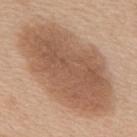| key | value |
|---|---|
| follow-up | no biopsy performed (imaged during a skin exam) |
| location | the mid back |
| tile lighting | white-light |
| diameter | about 13.5 mm |
| acquisition | ~15 mm tile from a whole-body skin photo |
| patient | male, aged around 60 |